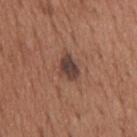Clinical summary: On the back. A male subject in their mid-60s. This is a white-light tile. This image is a 15 mm lesion crop taken from a total-body photograph. The recorded lesion diameter is about 3 mm.